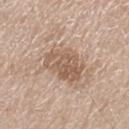| feature | finding |
|---|---|
| biopsy status | catalogued during a skin exam; not biopsied |
| illumination | white-light |
| size | about 5 mm |
| image source | ~15 mm tile from a whole-body skin photo |
| patient | male, aged around 80 |
| body site | the leg |
| TBP lesion metrics | a footprint of about 13 mm², an outline eccentricity of about 0.7 (0 = round, 1 = elongated), and a shape-asymmetry score of about 0.25 (0 = symmetric); about 10 CIELAB-L* units darker than the surrounding skin and a lesion-to-skin contrast of about 7 (normalized; higher = more distinct); a border-irregularity rating of about 3/10 and radial color variation of about 1.5; an automated nevus-likeness rating near 15 out of 100 and a detector confidence of about 100 out of 100 that the crop contains a lesion |